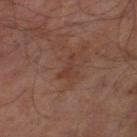Q: Was this lesion biopsied?
A: imaged on a skin check; not biopsied
Q: Illumination type?
A: cross-polarized
Q: Patient demographics?
A: male, in their mid-60s
Q: What kind of image is this?
A: total-body-photography crop, ~15 mm field of view
Q: What did automated image analysis measure?
A: a mean CIELAB color near L≈36 a*≈21 b*≈25, roughly 5 lightness units darker than nearby skin, and a normalized lesion–skin contrast near 5; a border-irregularity rating of about 6.5/10, a color-variation rating of about 0/10, and a peripheral color-asymmetry measure near 0
Q: Where on the body is the lesion?
A: the leg
Q: Lesion size?
A: ~3 mm (longest diameter)